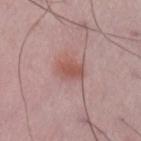Q: Was a biopsy performed?
A: total-body-photography surveillance lesion; no biopsy
Q: What is the anatomic site?
A: the arm
Q: What kind of image is this?
A: total-body-photography crop, ~15 mm field of view
Q: How large is the lesion?
A: ~3 mm (longest diameter)
Q: Patient demographics?
A: male, aged approximately 50
Q: Illumination type?
A: white-light illumination
Q: Automated lesion metrics?
A: a lesion area of about 5.5 mm², an outline eccentricity of about 0.6 (0 = round, 1 = elongated), and a symmetry-axis asymmetry near 0.2; a mean CIELAB color near L≈53 a*≈23 b*≈25, about 9 CIELAB-L* units darker than the surrounding skin, and a lesion-to-skin contrast of about 7.5 (normalized; higher = more distinct); border irregularity of about 1.5 on a 0–10 scale and internal color variation of about 2 on a 0–10 scale; an automated nevus-likeness rating near 80 out of 100 and a lesion-detection confidence of about 100/100